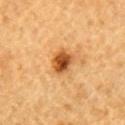Background:
Approximately 3 mm at its widest. Imaged with cross-polarized lighting. A 15 mm crop from a total-body photograph taken for skin-cancer surveillance. From the right upper arm. A male patient approximately 85 years of age or older.